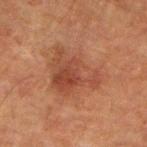Q: Was a biopsy performed?
A: total-body-photography surveillance lesion; no biopsy
Q: How was this image acquired?
A: 15 mm crop, total-body photography
Q: How large is the lesion?
A: ≈7.5 mm
Q: Who is the patient?
A: male, in their mid-60s
Q: Lesion location?
A: the left forearm
Q: What lighting was used for the tile?
A: cross-polarized illumination